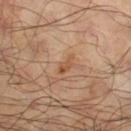notes=imaged on a skin check; not biopsied
acquisition=15 mm crop, total-body photography
patient=male, in their mid-60s
location=the leg
illumination=cross-polarized
lesion size=≈2.5 mm